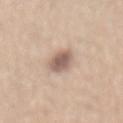Q: Was a biopsy performed?
A: imaged on a skin check; not biopsied
Q: How was this image acquired?
A: total-body-photography crop, ~15 mm field of view
Q: Who is the patient?
A: male, roughly 30 years of age
Q: Lesion size?
A: ≈3.5 mm
Q: Where on the body is the lesion?
A: the lower back
Q: Automated lesion metrics?
A: a nevus-likeness score of about 70/100 and a detector confidence of about 100 out of 100 that the crop contains a lesion
Q: What lighting was used for the tile?
A: white-light